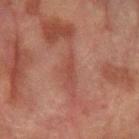Notes:
• workup — no biopsy performed (imaged during a skin exam)
• image source — 15 mm crop, total-body photography
• automated lesion analysis — a footprint of about 2.5 mm², an outline eccentricity of about 0.95 (0 = round, 1 = elongated), and two-axis asymmetry of about 0.55; an average lesion color of about L≈38 a*≈23 b*≈24 (CIELAB), roughly 6 lightness units darker than nearby skin, and a normalized lesion–skin contrast near 5.5; border irregularity of about 6 on a 0–10 scale and internal color variation of about 0 on a 0–10 scale
• location — the left forearm
• subject — male, in their mid- to late 70s
• lesion diameter — ~3 mm (longest diameter)
• tile lighting — cross-polarized